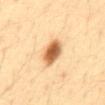Clinical impression:
This lesion was catalogued during total-body skin photography and was not selected for biopsy.
Acquisition and patient details:
Approximately 4 mm at its widest. This is a cross-polarized tile. The total-body-photography lesion software estimated a footprint of about 8.5 mm² and a shape-asymmetry score of about 0.15 (0 = symmetric). And it measured a lesion color around L≈59 a*≈20 b*≈38 in CIELAB, about 17 CIELAB-L* units darker than the surrounding skin, and a normalized border contrast of about 10.5. It also reported an automated nevus-likeness rating near 100 out of 100. Cropped from a whole-body photographic skin survey; the tile spans about 15 mm. A male subject, approximately 35 years of age. The lesion is located on the abdomen.